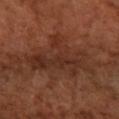Q: Was this lesion biopsied?
A: catalogued during a skin exam; not biopsied
Q: What are the patient's age and sex?
A: female, in their mid- to late 60s
Q: Lesion location?
A: the arm
Q: How was this image acquired?
A: 15 mm crop, total-body photography